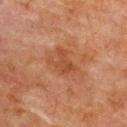This lesion was catalogued during total-body skin photography and was not selected for biopsy. The lesion is located on the upper back. An algorithmic analysis of the crop reported a normalized lesion–skin contrast near 6. And it measured an automated nevus-likeness rating near 0 out of 100 and a lesion-detection confidence of about 100/100. This is a cross-polarized tile. The patient is a female in their 80s. About 3.5 mm across. This image is a 15 mm lesion crop taken from a total-body photograph.